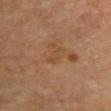{"biopsy_status": "not biopsied; imaged during a skin examination", "lesion_size": {"long_diameter_mm_approx": 2.5}, "image": {"source": "total-body photography crop", "field_of_view_mm": 15}, "patient": {"sex": "female", "age_approx": 70}, "site": "chest", "lighting": "cross-polarized"}